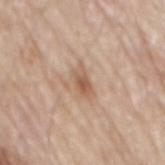{
  "biopsy_status": "not biopsied; imaged during a skin examination",
  "lesion_size": {
    "long_diameter_mm_approx": 3.5
  },
  "site": "mid back",
  "lighting": "white-light",
  "image": {
    "source": "total-body photography crop",
    "field_of_view_mm": 15
  },
  "patient": {
    "sex": "male",
    "age_approx": 80
  }
}